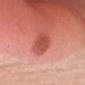Notes:
• lesion size — ≈3.5 mm
• image source — ~15 mm crop, total-body skin-cancer survey
• automated lesion analysis — a footprint of about 6.5 mm², a shape eccentricity near 0.75, and a shape-asymmetry score of about 0.15 (0 = symmetric); a lesion color around L≈52 a*≈34 b*≈32 in CIELAB; a nevus-likeness score of about 15/100 and lesion-presence confidence of about 100/100
• patient — female, approximately 45 years of age
• anatomic site — the head or neck
• illumination — white-light illumination